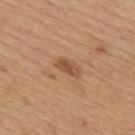  biopsy_status: not biopsied; imaged during a skin examination
  site: back
  image:
    source: total-body photography crop
    field_of_view_mm: 15
  patient:
    sex: female
    age_approx: 30